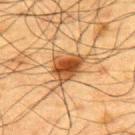follow-up = catalogued during a skin exam; not biopsied